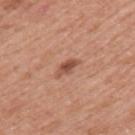| field | value |
|---|---|
| acquisition | total-body-photography crop, ~15 mm field of view |
| anatomic site | the upper back |
| patient | female, roughly 40 years of age |